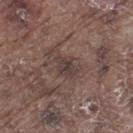Recorded during total-body skin imaging; not selected for excision or biopsy.
A 15 mm close-up extracted from a 3D total-body photography capture.
The subject is a male about 75 years old.
From the left thigh.